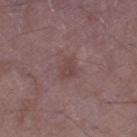<record>
<biopsy_status>not biopsied; imaged during a skin examination</biopsy_status>
<image>
  <source>total-body photography crop</source>
  <field_of_view_mm>15</field_of_view_mm>
</image>
<lighting>white-light</lighting>
<site>right thigh</site>
<automated_metrics>
  <area_mm2_approx>4.0</area_mm2_approx>
  <eccentricity>0.7</eccentricity>
  <shape_asymmetry>0.3</shape_asymmetry>
  <nevus_likeness_0_100>0</nevus_likeness_0_100>
  <lesion_detection_confidence_0_100>100</lesion_detection_confidence_0_100>
</automated_metrics>
<patient>
  <sex>male</sex>
  <age_approx>50</age_approx>
</patient>
<lesion_size>
  <long_diameter_mm_approx>3.0</long_diameter_mm_approx>
</lesion_size>
</record>